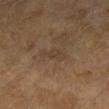{"biopsy_status": "not biopsied; imaged during a skin examination", "site": "left forearm", "patient": {"sex": "female", "age_approx": 60}, "image": {"source": "total-body photography crop", "field_of_view_mm": 15}}